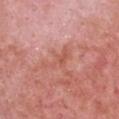Context:
Approximately 4.5 mm at its widest. A 15 mm crop from a total-body photograph taken for skin-cancer surveillance. Captured under white-light illumination. An algorithmic analysis of the crop reported a border-irregularity index near 6/10, a color-variation rating of about 1.5/10, and radial color variation of about 0.5. It also reported a nevus-likeness score of about 0/100 and a lesion-detection confidence of about 100/100. On the chest. A female subject aged 38–42.